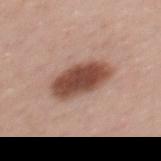This lesion was catalogued during total-body skin photography and was not selected for biopsy. A female subject aged 33 to 37. An algorithmic analysis of the crop reported an area of roughly 15 mm², an eccentricity of roughly 0.85, and two-axis asymmetry of about 0.15. The software also gave a mean CIELAB color near L≈48 a*≈22 b*≈27, a lesion–skin lightness drop of about 17, and a normalized border contrast of about 12. And it measured a nevus-likeness score of about 95/100. From the upper back. Measured at roughly 6 mm in maximum diameter. The tile uses white-light illumination. A close-up tile cropped from a whole-body skin photograph, about 15 mm across.